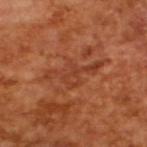Case summary:
– image source · 15 mm crop, total-body photography
– image-analysis metrics · a border-irregularity index near 8.5/10, internal color variation of about 2.5 on a 0–10 scale, and peripheral color asymmetry of about 1
– subject · male, in their mid- to late 60s